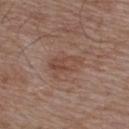No biopsy was performed on this lesion — it was imaged during a full skin examination and was not determined to be concerning. A roughly 15 mm field-of-view crop from a total-body skin photograph. A male patient, roughly 55 years of age. The lesion is on the upper back.